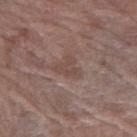Impression:
The lesion was photographed on a routine skin check and not biopsied; there is no pathology result.
Context:
A female subject aged approximately 65. The lesion is on the right forearm. A lesion tile, about 15 mm wide, cut from a 3D total-body photograph.